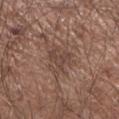biopsy_status: not biopsied; imaged during a skin examination
lesion_size:
  long_diameter_mm_approx: 4.0
site: right upper arm
patient:
  sex: male
  age_approx: 65
automated_metrics:
  cielab_L: 43
  cielab_a: 17
  cielab_b: 24
  vs_skin_darker_L: 7.0
  border_irregularity_0_10: 7.5
  color_variation_0_10: 2.0
  peripheral_color_asymmetry: 1.0
  nevus_likeness_0_100: 0
  lesion_detection_confidence_0_100: 75
image:
  source: total-body photography crop
  field_of_view_mm: 15